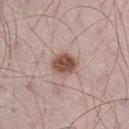Clinical impression:
This lesion was catalogued during total-body skin photography and was not selected for biopsy.
Background:
Captured under white-light illumination. Located on the leg. Cropped from a whole-body photographic skin survey; the tile spans about 15 mm. About 3.5 mm across. A male subject in their 70s.